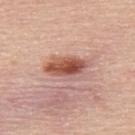* workup · catalogued during a skin exam; not biopsied
* acquisition · total-body-photography crop, ~15 mm field of view
* lighting · white-light
* location · the upper back
* patient · male, approximately 60 years of age
* diameter · about 5 mm
* automated lesion analysis · an area of roughly 11 mm² and a symmetry-axis asymmetry near 0.25; an automated nevus-likeness rating near 100 out of 100